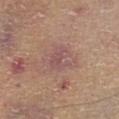Image and clinical context: The lesion's longest dimension is about 4 mm. On the front of the torso. A male subject aged 83 or over. Imaged with white-light lighting. This image is a 15 mm lesion crop taken from a total-body photograph.